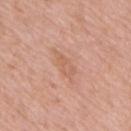Clinical impression: Part of a total-body skin-imaging series; this lesion was reviewed on a skin check and was not flagged for biopsy. Image and clinical context: A close-up tile cropped from a whole-body skin photograph, about 15 mm across. The patient is a male roughly 60 years of age. The lesion is located on the mid back.